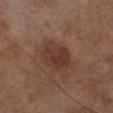Clinical impression: The lesion was tiled from a total-body skin photograph and was not biopsied. Clinical summary: Measured at roughly 4.5 mm in maximum diameter. The lesion-visualizer software estimated an average lesion color of about L≈34 a*≈19 b*≈24 (CIELAB), about 8 CIELAB-L* units darker than the surrounding skin, and a normalized lesion–skin contrast near 7. The software also gave a border-irregularity rating of about 2/10, a within-lesion color-variation index near 3/10, and radial color variation of about 1. The analysis additionally found a nevus-likeness score of about 60/100 and a detector confidence of about 100 out of 100 that the crop contains a lesion. A male subject approximately 65 years of age. A 15 mm crop from a total-body photograph taken for skin-cancer surveillance. This is a cross-polarized tile.